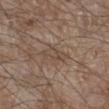Assessment:
Captured during whole-body skin photography for melanoma surveillance; the lesion was not biopsied.
Background:
Approximately 3.5 mm at its widest. Cropped from a total-body skin-imaging series; the visible field is about 15 mm. Located on the left lower leg. The total-body-photography lesion software estimated a footprint of about 4.5 mm², an eccentricity of roughly 0.9, and two-axis asymmetry of about 0.25. It also reported an average lesion color of about L≈46 a*≈14 b*≈24 (CIELAB) and a lesion-to-skin contrast of about 5 (normalized; higher = more distinct). The analysis additionally found a within-lesion color-variation index near 2.5/10. And it measured an automated nevus-likeness rating near 0 out of 100. This is a white-light tile. A male subject about 65 years old.